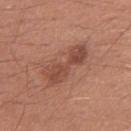{
  "biopsy_status": "not biopsied; imaged during a skin examination"
}